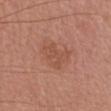Clinical impression:
Part of a total-body skin-imaging series; this lesion was reviewed on a skin check and was not flagged for biopsy.
Context:
The patient is a male roughly 65 years of age. Automated image analysis of the tile measured a lesion area of about 8 mm², a shape eccentricity near 0.55, and a symmetry-axis asymmetry near 0.35. The analysis additionally found border irregularity of about 4 on a 0–10 scale, a within-lesion color-variation index near 2/10, and a peripheral color-asymmetry measure near 0.5. The analysis additionally found a nevus-likeness score of about 0/100 and a lesion-detection confidence of about 100/100. A lesion tile, about 15 mm wide, cut from a 3D total-body photograph. The lesion is on the chest. Captured under white-light illumination. The lesion's longest dimension is about 3.5 mm.